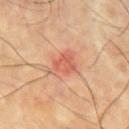Measured at roughly 4 mm in maximum diameter.
A lesion tile, about 15 mm wide, cut from a 3D total-body photograph.
Located on the chest.
The total-body-photography lesion software estimated a border-irregularity index near 4/10, internal color variation of about 4 on a 0–10 scale, and a peripheral color-asymmetry measure near 1.5. The software also gave an automated nevus-likeness rating near 0 out of 100 and lesion-presence confidence of about 100/100.
Captured under cross-polarized illumination.